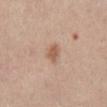{
  "biopsy_status": "not biopsied; imaged during a skin examination",
  "lighting": "white-light",
  "patient": {
    "sex": "male",
    "age_approx": 40
  },
  "site": "front of the torso",
  "image": {
    "source": "total-body photography crop",
    "field_of_view_mm": 15
  },
  "automated_metrics": {
    "area_mm2_approx": 3.5,
    "shape_asymmetry": 0.2,
    "nevus_likeness_0_100": 70,
    "lesion_detection_confidence_0_100": 100
  },
  "lesion_size": {
    "long_diameter_mm_approx": 2.5
  }
}